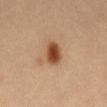Case summary:
- biopsy status — total-body-photography surveillance lesion; no biopsy
- imaging modality — 15 mm crop, total-body photography
- patient — male, aged approximately 40
- tile lighting — cross-polarized illumination
- lesion size — about 3 mm
- body site — the abdomen